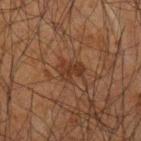Captured during whole-body skin photography for melanoma surveillance; the lesion was not biopsied.
A 15 mm close-up extracted from a 3D total-body photography capture.
A male subject, in their mid- to late 60s.
Located on the left forearm.
Measured at roughly 3 mm in maximum diameter.